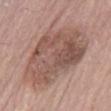Recorded during total-body skin imaging; not selected for excision or biopsy. From the abdomen. A 15 mm close-up extracted from a 3D total-body photography capture. A male subject aged around 70.